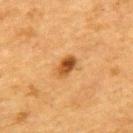biopsy status: imaged on a skin check; not biopsied | subject: female, aged around 55 | anatomic site: the upper back | image: ~15 mm crop, total-body skin-cancer survey | illumination: cross-polarized | lesion diameter: about 3 mm.